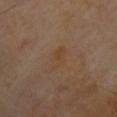Clinical impression:
Captured during whole-body skin photography for melanoma surveillance; the lesion was not biopsied.
Background:
About 3.5 mm across. A lesion tile, about 15 mm wide, cut from a 3D total-body photograph. The tile uses cross-polarized illumination. On the chest. The subject is a male about 65 years old.